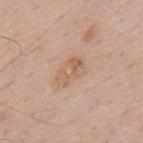notes: imaged on a skin check; not biopsied
acquisition: ~15 mm tile from a whole-body skin photo
location: the upper back
patient: male, roughly 70 years of age
lighting: white-light illumination
automated metrics: an area of roughly 8.5 mm² and an outline eccentricity of about 0.8 (0 = round, 1 = elongated); a border-irregularity index near 2.5/10 and a within-lesion color-variation index near 5/10; a nevus-likeness score of about 0/100 and lesion-presence confidence of about 100/100
lesion size: ~4 mm (longest diameter)